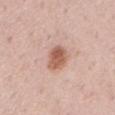notes: imaged on a skin check; not biopsied | acquisition: ~15 mm crop, total-body skin-cancer survey | diameter: about 3.5 mm | location: the mid back | illumination: white-light | automated lesion analysis: a lesion area of about 7 mm², an outline eccentricity of about 0.75 (0 = round, 1 = elongated), and two-axis asymmetry of about 0.15; border irregularity of about 2 on a 0–10 scale, a color-variation rating of about 4.5/10, and a peripheral color-asymmetry measure near 1.5 | subject: male, aged approximately 70.